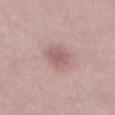The lesion was tiled from a total-body skin photograph and was not biopsied. A male patient aged 28–32. A 15 mm crop from a total-body photograph taken for skin-cancer surveillance. The total-body-photography lesion software estimated a mean CIELAB color near L≈58 a*≈21 b*≈19, roughly 10 lightness units darker than nearby skin, and a normalized border contrast of about 6.5. The software also gave a lesion-detection confidence of about 100/100. The lesion's longest dimension is about 4 mm. Imaged with white-light lighting.